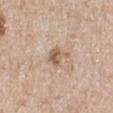Findings:
- workup — total-body-photography surveillance lesion; no biopsy
- site — the left lower leg
- lesion size — ~3 mm (longest diameter)
- acquisition — total-body-photography crop, ~15 mm field of view
- patient — female, aged approximately 70
- tile lighting — white-light